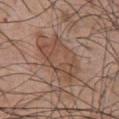This is a white-light tile. An algorithmic analysis of the crop reported an area of roughly 19 mm² and an outline eccentricity of about 0.75 (0 = round, 1 = elongated). And it measured a border-irregularity rating of about 3/10, a color-variation rating of about 4/10, and radial color variation of about 1.5. And it measured a classifier nevus-likeness of about 5/100. Cropped from a whole-body photographic skin survey; the tile spans about 15 mm. Located on the chest. A male subject aged approximately 45.